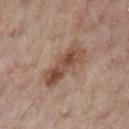Q: Was this lesion biopsied?
A: no biopsy performed (imaged during a skin exam)
Q: Who is the patient?
A: female, about 55 years old
Q: What is the imaging modality?
A: ~15 mm crop, total-body skin-cancer survey
Q: What is the anatomic site?
A: the arm